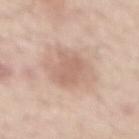biopsy status: imaged on a skin check; not biopsied | lighting: white-light | image: 15 mm crop, total-body photography | lesion diameter: about 4.5 mm | patient: male, aged approximately 55 | site: the mid back.